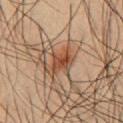The lesion was photographed on a routine skin check and not biopsied; there is no pathology result.
This image is a 15 mm lesion crop taken from a total-body photograph.
The lesion is on the chest.
A male patient, aged 58 to 62.